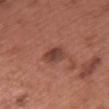Clinical impression: Captured during whole-body skin photography for melanoma surveillance; the lesion was not biopsied. Context: This is a white-light tile. A 15 mm close-up extracted from a 3D total-body photography capture. The total-body-photography lesion software estimated a shape eccentricity near 0.75. The analysis additionally found a normalized border contrast of about 8. The lesion is located on the chest. Approximately 3 mm at its widest. The subject is a female aged 58 to 62.